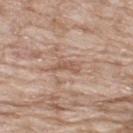Notes:
– follow-up — catalogued during a skin exam; not biopsied
– automated lesion analysis — an area of roughly 4 mm² and two-axis asymmetry of about 0.35; a lesion color around L≈56 a*≈19 b*≈29 in CIELAB and roughly 9 lightness units darker than nearby skin; a border-irregularity rating of about 4/10, a within-lesion color-variation index near 1.5/10, and radial color variation of about 0; a classifier nevus-likeness of about 0/100 and a detector confidence of about 70 out of 100 that the crop contains a lesion
– site — the upper back
– patient — male, about 65 years old
– tile lighting — white-light illumination
– size — about 3.5 mm
– image source — ~15 mm tile from a whole-body skin photo